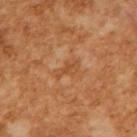Clinical summary:
A roughly 15 mm field-of-view crop from a total-body skin photograph. A male subject, roughly 65 years of age. The tile uses cross-polarized illumination.